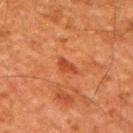- biopsy status — imaged on a skin check; not biopsied
- patient — male, aged around 60
- site — the arm
- imaging modality — total-body-photography crop, ~15 mm field of view